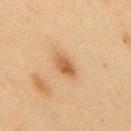Recorded during total-body skin imaging; not selected for excision or biopsy. A male subject, in their mid-40s. Cropped from a whole-body photographic skin survey; the tile spans about 15 mm. On the upper back. The total-body-photography lesion software estimated an eccentricity of roughly 0.8 and two-axis asymmetry of about 0.2. The tile uses cross-polarized illumination. About 3.5 mm across.